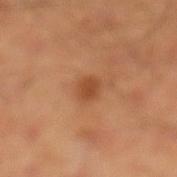Measured at roughly 2.5 mm in maximum diameter. The lesion is located on the leg. Imaged with cross-polarized lighting. A male patient, in their mid-50s. A lesion tile, about 15 mm wide, cut from a 3D total-body photograph.